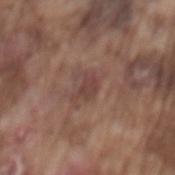workup=catalogued during a skin exam; not biopsied
subject=male, about 75 years old
location=the mid back
imaging modality=15 mm crop, total-body photography